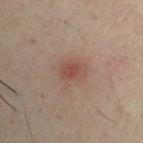  biopsy_status: not biopsied; imaged during a skin examination
  image:
    source: total-body photography crop
    field_of_view_mm: 15
  lesion_size:
    long_diameter_mm_approx: 3.0
  patient:
    sex: male
    age_approx: 30
  site: right upper arm